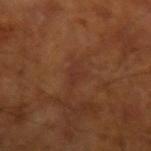No biopsy was performed on this lesion — it was imaged during a full skin examination and was not determined to be concerning. A male patient, roughly 65 years of age. An algorithmic analysis of the crop reported a lesion area of about 3 mm² and a shape-asymmetry score of about 0.65 (0 = symmetric). And it measured a lesion color around L≈30 a*≈22 b*≈26 in CIELAB, a lesion–skin lightness drop of about 4, and a normalized lesion–skin contrast near 5. The analysis additionally found a border-irregularity rating of about 6.5/10 and a color-variation rating of about 0/10. The lesion's longest dimension is about 2.5 mm. A roughly 15 mm field-of-view crop from a total-body skin photograph. On the arm.